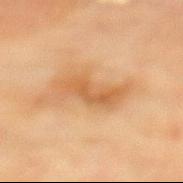Assessment:
The lesion was tiled from a total-body skin photograph and was not biopsied.
Background:
A lesion tile, about 15 mm wide, cut from a 3D total-body photograph. On the mid back. About 6 mm across. Captured under cross-polarized illumination. A female subject aged approximately 80.